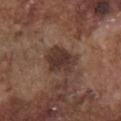workup: catalogued during a skin exam; not biopsied | image: total-body-photography crop, ~15 mm field of view | automated metrics: a footprint of about 9 mm² and an eccentricity of roughly 0.65; an average lesion color of about L≈34 a*≈17 b*≈22 (CIELAB), about 11 CIELAB-L* units darker than the surrounding skin, and a lesion-to-skin contrast of about 10 (normalized; higher = more distinct) | site: the chest | lesion size: ≈4 mm | illumination: white-light | patient: male, in their mid-70s.